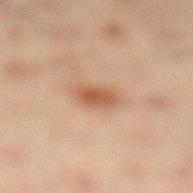biopsy status: imaged on a skin check; not biopsied | tile lighting: cross-polarized | subject: male, aged 43 to 47 | site: the leg | image: ~15 mm tile from a whole-body skin photo.